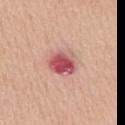{"biopsy_status": "not biopsied; imaged during a skin examination", "image": {"source": "total-body photography crop", "field_of_view_mm": 15}, "patient": {"sex": "male", "age_approx": 65}, "automated_metrics": {"shape_asymmetry": 0.2, "cielab_L": 56, "cielab_a": 32, "cielab_b": 23, "vs_skin_contrast_norm": 10.0, "nevus_likeness_0_100": 0, "lesion_detection_confidence_0_100": 100}, "lesion_size": {"long_diameter_mm_approx": 3.5}, "site": "chest"}